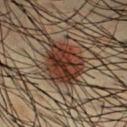Clinical impression:
The lesion was photographed on a routine skin check and not biopsied; there is no pathology result.
Background:
The lesion's longest dimension is about 5 mm. A region of skin cropped from a whole-body photographic capture, roughly 15 mm wide. Imaged with cross-polarized lighting. The lesion-visualizer software estimated a border-irregularity index near 2.5/10. A male subject approximately 35 years of age. The lesion is on the chest.